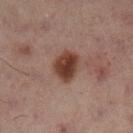  biopsy_status: not biopsied; imaged during a skin examination
  lighting: cross-polarized
  site: left leg
  automated_metrics:
    area_mm2_approx: 8.0
    shape_asymmetry: 0.15
    nevus_likeness_0_100: 100
  lesion_size:
    long_diameter_mm_approx: 4.0
  image:
    source: total-body photography crop
    field_of_view_mm: 15
  patient:
    sex: female
    age_approx: 55